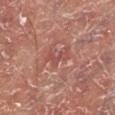biopsy status = catalogued during a skin exam; not biopsied
tile lighting = white-light
acquisition = ~15 mm crop, total-body skin-cancer survey
location = the left lower leg
subject = male, in their mid- to late 50s
size = ≈3.5 mm
automated lesion analysis = an average lesion color of about L≈51 a*≈27 b*≈26 (CIELAB), a lesion–skin lightness drop of about 7, and a normalized lesion–skin contrast near 5; a border-irregularity index near 7.5/10, a within-lesion color-variation index near 0/10, and peripheral color asymmetry of about 0; a classifier nevus-likeness of about 0/100 and a detector confidence of about 65 out of 100 that the crop contains a lesion